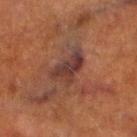Part of a total-body skin-imaging series; this lesion was reviewed on a skin check and was not flagged for biopsy.
A 15 mm crop from a total-body photograph taken for skin-cancer surveillance.
An algorithmic analysis of the crop reported a nevus-likeness score of about 0/100 and lesion-presence confidence of about 85/100.
The recorded lesion diameter is about 5 mm.
From the leg.
The subject is a male roughly 70 years of age.